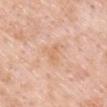  biopsy_status: not biopsied; imaged during a skin examination
  lesion_size:
    long_diameter_mm_approx: 3.0
  lighting: white-light
  site: left upper arm
  automated_metrics:
    eccentricity: 0.75
    shape_asymmetry: 0.45
  image:
    source: total-body photography crop
    field_of_view_mm: 15
  patient:
    sex: male
    age_approx: 55